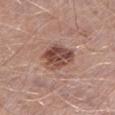{
  "biopsy_status": "not biopsied; imaged during a skin examination",
  "lighting": "white-light",
  "patient": {
    "sex": "male",
    "age_approx": 50
  },
  "site": "left lower leg",
  "image": {
    "source": "total-body photography crop",
    "field_of_view_mm": 15
  }
}